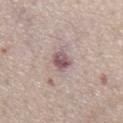biopsy_status: not biopsied; imaged during a skin examination
image:
  source: total-body photography crop
  field_of_view_mm: 15
patient:
  sex: male
  age_approx: 75
lighting: white-light
automated_metrics:
  border_irregularity_0_10: 2.5
  color_variation_0_10: 4.5
  peripheral_color_asymmetry: 1.5
site: chest
lesion_size:
  long_diameter_mm_approx: 3.0